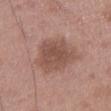biopsy status: total-body-photography surveillance lesion; no biopsy
location: the left thigh
image: ~15 mm crop, total-body skin-cancer survey
size: ≈6 mm
patient: male, about 50 years old
tile lighting: white-light illumination